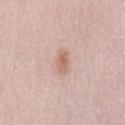notes: catalogued during a skin exam; not biopsied
subject: female, in their mid- to late 60s
anatomic site: the lower back
image: total-body-photography crop, ~15 mm field of view